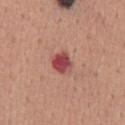biopsy status — imaged on a skin check; not biopsied
acquisition — ~15 mm tile from a whole-body skin photo
lesion size — ≈3 mm
automated lesion analysis — internal color variation of about 3 on a 0–10 scale and a peripheral color-asymmetry measure near 1
tile lighting — white-light
patient — male, approximately 45 years of age
body site — the chest